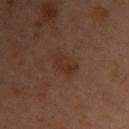{
  "biopsy_status": "not biopsied; imaged during a skin examination",
  "image": {
    "source": "total-body photography crop",
    "field_of_view_mm": 15
  },
  "lighting": "cross-polarized",
  "patient": {
    "sex": "female",
    "age_approx": 55
  },
  "lesion_size": {
    "long_diameter_mm_approx": 3.5
  },
  "site": "arm"
}